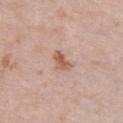No biopsy was performed on this lesion — it was imaged during a full skin examination and was not determined to be concerning. A female subject, roughly 40 years of age. A lesion tile, about 15 mm wide, cut from a 3D total-body photograph. Imaged with white-light lighting. The lesion is located on the front of the torso.